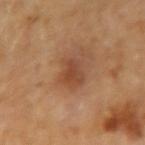Located on the arm. A female subject aged 58–62. The lesion-visualizer software estimated an average lesion color of about L≈45 a*≈23 b*≈33 (CIELAB), a lesion–skin lightness drop of about 8, and a lesion-to-skin contrast of about 6.5 (normalized; higher = more distinct). The analysis additionally found an automated nevus-likeness rating near 55 out of 100 and lesion-presence confidence of about 100/100. Captured under cross-polarized illumination. About 3.5 mm across. A region of skin cropped from a whole-body photographic capture, roughly 15 mm wide.